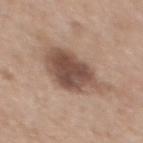Case summary:
* notes — total-body-photography surveillance lesion; no biopsy
* anatomic site — the upper back
* image — ~15 mm tile from a whole-body skin photo
* patient — female, roughly 50 years of age
* tile lighting — white-light